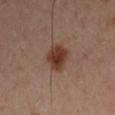The lesion was photographed on a routine skin check and not biopsied; there is no pathology result. A 15 mm crop from a total-body photograph taken for skin-cancer surveillance. A male subject aged 43–47. Located on the right upper arm.